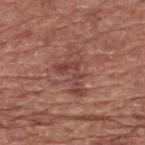biopsy status: total-body-photography surveillance lesion; no biopsy | lesion size: ~4.5 mm (longest diameter) | TBP lesion metrics: a lesion area of about 9 mm² and a shape-asymmetry score of about 0.7 (0 = symmetric); an average lesion color of about L≈44 a*≈24 b*≈24 (CIELAB) and a normalized border contrast of about 6; a within-lesion color-variation index near 4/10 and radial color variation of about 1 | anatomic site: the upper back | subject: male, aged approximately 75 | tile lighting: white-light | imaging modality: ~15 mm tile from a whole-body skin photo.